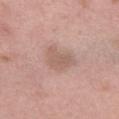{"automated_metrics": {"area_mm2_approx": 6.5, "eccentricity": 0.75, "shape_asymmetry": 0.4, "peripheral_color_asymmetry": 0.5, "nevus_likeness_0_100": 0, "lesion_detection_confidence_0_100": 100}, "lighting": "white-light", "site": "left thigh", "image": {"source": "total-body photography crop", "field_of_view_mm": 15}, "lesion_size": {"long_diameter_mm_approx": 3.5}, "patient": {"sex": "female", "age_approx": 50}}